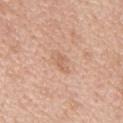A 15 mm crop from a total-body photograph taken for skin-cancer surveillance.
Captured under white-light illumination.
The lesion's longest dimension is about 3 mm.
A male subject in their 50s.
The lesion is located on the back.